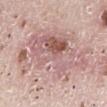Q: Was a biopsy performed?
A: imaged on a skin check; not biopsied
Q: Automated lesion metrics?
A: border irregularity of about 4.5 on a 0–10 scale and internal color variation of about 9.5 on a 0–10 scale; a classifier nevus-likeness of about 0/100
Q: How was this image acquired?
A: ~15 mm tile from a whole-body skin photo
Q: Who is the patient?
A: female, in their mid- to late 40s
Q: Where on the body is the lesion?
A: the left lower leg
Q: Lesion size?
A: ~12 mm (longest diameter)